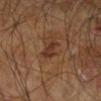biopsy_status: not biopsied; imaged during a skin examination
patient:
  sex: male
  age_approx: 60
image:
  source: total-body photography crop
  field_of_view_mm: 15
lesion_size:
  long_diameter_mm_approx: 3.0
automated_metrics:
  area_mm2_approx: 3.5
  eccentricity: 0.9
  shape_asymmetry: 0.3
  border_irregularity_0_10: 3.0
  nevus_likeness_0_100: 15
  lesion_detection_confidence_0_100: 100
site: right leg